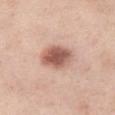Clinical impression: This lesion was catalogued during total-body skin photography and was not selected for biopsy. Clinical summary: A 15 mm close-up extracted from a 3D total-body photography capture. Approximately 4.5 mm at its widest. On the left thigh. The subject is a female aged around 55. An algorithmic analysis of the crop reported a lesion area of about 11 mm² and a shape-asymmetry score of about 0.15 (0 = symmetric). And it measured a border-irregularity rating of about 1.5/10 and a peripheral color-asymmetry measure near 1.5. The analysis additionally found an automated nevus-likeness rating near 95 out of 100 and a detector confidence of about 100 out of 100 that the crop contains a lesion. The tile uses white-light illumination.